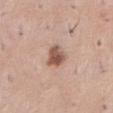{"biopsy_status": "not biopsied; imaged during a skin examination", "patient": {"sex": "female", "age_approx": 45}, "lighting": "white-light", "site": "abdomen", "image": {"source": "total-body photography crop", "field_of_view_mm": 15}, "automated_metrics": {"cielab_L": 54, "cielab_a": 20, "cielab_b": 27, "vs_skin_darker_L": 14.0, "vs_skin_contrast_norm": 9.5, "border_irregularity_0_10": 2.5, "peripheral_color_asymmetry": 1.5, "lesion_detection_confidence_0_100": 100}}